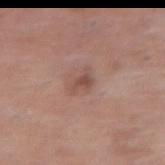| feature | finding |
|---|---|
| follow-up | catalogued during a skin exam; not biopsied |
| subject | female, approximately 65 years of age |
| image-analysis metrics | a lesion area of about 3 mm², an eccentricity of roughly 0.8, and two-axis asymmetry of about 0.55; border irregularity of about 5.5 on a 0–10 scale, internal color variation of about 1.5 on a 0–10 scale, and radial color variation of about 0.5; a nevus-likeness score of about 0/100 and a detector confidence of about 100 out of 100 that the crop contains a lesion |
| lesion size | ≈3 mm |
| acquisition | total-body-photography crop, ~15 mm field of view |
| tile lighting | white-light illumination |
| location | the right thigh |